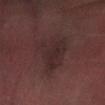Q: Was this lesion biopsied?
A: imaged on a skin check; not biopsied
Q: Patient demographics?
A: male, aged around 65
Q: What is the lesion's diameter?
A: ≈6 mm
Q: What did automated image analysis measure?
A: a within-lesion color-variation index near 2.5/10 and a peripheral color-asymmetry measure near 1; a classifier nevus-likeness of about 0/100
Q: How was the tile lit?
A: cross-polarized illumination
Q: Where on the body is the lesion?
A: the arm
Q: What kind of image is this?
A: ~15 mm tile from a whole-body skin photo